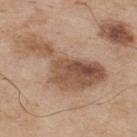  biopsy_status: not biopsied; imaged during a skin examination
  automated_metrics:
    cielab_L: 52
    cielab_a: 19
    cielab_b: 30
    vs_skin_darker_L: 13.0
    vs_skin_contrast_norm: 9.0
    border_irregularity_0_10: 8.0
    color_variation_0_10: 6.5
    peripheral_color_asymmetry: 2.5
  site: upper back
  lesion_size:
    long_diameter_mm_approx: 10.0
  patient:
    sex: male
    age_approx: 75
  image:
    source: total-body photography crop
    field_of_view_mm: 15